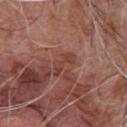Findings:
* biopsy status · imaged on a skin check; not biopsied
* image source · ~15 mm crop, total-body skin-cancer survey
* patient · male, in their mid-70s
* body site · the chest